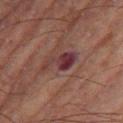Impression: The lesion was photographed on a routine skin check and not biopsied; there is no pathology result. Context: A lesion tile, about 15 mm wide, cut from a 3D total-body photograph. The lesion is located on the right thigh. This is a cross-polarized tile. About 4 mm across. The subject is a male aged 68 to 72.